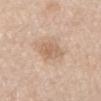| feature | finding |
|---|---|
| follow-up | no biopsy performed (imaged during a skin exam) |
| acquisition | ~15 mm crop, total-body skin-cancer survey |
| subject | female, aged 63 to 67 |
| diameter | about 3 mm |
| anatomic site | the mid back |